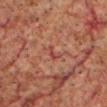No biopsy was performed on this lesion — it was imaged during a full skin examination and was not determined to be concerning. The lesion's longest dimension is about 2.5 mm. From the head or neck. Captured under cross-polarized illumination. Cropped from a total-body skin-imaging series; the visible field is about 15 mm. A male subject, about 60 years old. Automated image analysis of the tile measured a lesion area of about 2.5 mm² and an outline eccentricity of about 0.9 (0 = round, 1 = elongated). It also reported a lesion–skin lightness drop of about 6 and a normalized border contrast of about 5.5. The analysis additionally found a border-irregularity rating of about 5.5/10, a within-lesion color-variation index near 0/10, and peripheral color asymmetry of about 0. It also reported a detector confidence of about 90 out of 100 that the crop contains a lesion.